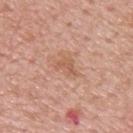{
  "biopsy_status": "not biopsied; imaged during a skin examination",
  "image": {
    "source": "total-body photography crop",
    "field_of_view_mm": 15
  },
  "lighting": "white-light",
  "lesion_size": {
    "long_diameter_mm_approx": 3.0
  },
  "site": "upper back",
  "patient": {
    "sex": "male",
    "age_approx": 75
  }
}